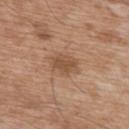| key | value |
|---|---|
| image-analysis metrics | a lesion color around L≈51 a*≈20 b*≈32 in CIELAB, a lesion–skin lightness drop of about 9, and a lesion-to-skin contrast of about 6.5 (normalized; higher = more distinct); lesion-presence confidence of about 100/100 |
| imaging modality | ~15 mm crop, total-body skin-cancer survey |
| lesion size | ≈3 mm |
| body site | the chest |
| subject | male, aged 58 to 62 |